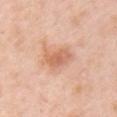<case>
  <biopsy_status>not biopsied; imaged during a skin examination</biopsy_status>
  <patient>
    <sex>female</sex>
    <age_approx>65</age_approx>
  </patient>
  <site>chest</site>
  <lesion_size>
    <long_diameter_mm_approx>3.5</long_diameter_mm_approx>
  </lesion_size>
  <image>
    <source>total-body photography crop</source>
    <field_of_view_mm>15</field_of_view_mm>
  </image>
  <lighting>white-light</lighting>
</case>